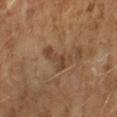Notes:
* notes — no biopsy performed (imaged during a skin exam)
* automated metrics — an eccentricity of roughly 0.9 and a shape-asymmetry score of about 0.5 (0 = symmetric); a mean CIELAB color near L≈39 a*≈16 b*≈28, a lesion–skin lightness drop of about 7, and a normalized border contrast of about 6; lesion-presence confidence of about 95/100
* location — the left forearm
* subject — male, aged around 60
* lesion size — about 4.5 mm
* image source — total-body-photography crop, ~15 mm field of view
* illumination — cross-polarized